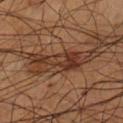image source = ~15 mm tile from a whole-body skin photo
subject = male, about 55 years old
body site = the left lower leg
size = ≈8 mm